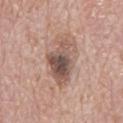biopsy status: imaged on a skin check; not biopsied
lighting: white-light illumination
subject: male, approximately 70 years of age
diameter: about 6.5 mm
image: 15 mm crop, total-body photography
anatomic site: the mid back